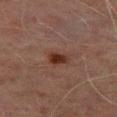follow-up = no biopsy performed (imaged during a skin exam); location = the right thigh; image = total-body-photography crop, ~15 mm field of view; lesion diameter = about 2.5 mm; automated lesion analysis = an area of roughly 4 mm², a shape eccentricity near 0.75, and two-axis asymmetry of about 0.2; tile lighting = cross-polarized; subject = male, roughly 70 years of age.